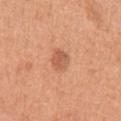Assessment: This lesion was catalogued during total-body skin photography and was not selected for biopsy. Background: The lesion is located on the left upper arm. A female patient approximately 35 years of age. A 15 mm close-up extracted from a 3D total-body photography capture.